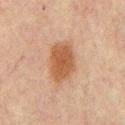Notes:
- image — ~15 mm crop, total-body skin-cancer survey
- anatomic site — the mid back
- lesion diameter — ≈5 mm
- subject — male, aged approximately 65
- image-analysis metrics — border irregularity of about 2 on a 0–10 scale, a color-variation rating of about 2.5/10, and radial color variation of about 0.5
- lighting — cross-polarized illumination